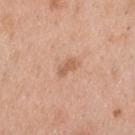notes: catalogued during a skin exam; not biopsied
location: the upper back
size: ≈2.5 mm
image source: ~15 mm crop, total-body skin-cancer survey
patient: male, approximately 40 years of age